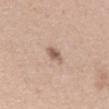biopsy_status: not biopsied; imaged during a skin examination
site: right forearm
patient:
  sex: female
  age_approx: 25
image:
  source: total-body photography crop
  field_of_view_mm: 15